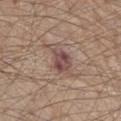notes — total-body-photography surveillance lesion; no biopsy | automated lesion analysis — a lesion area of about 7.5 mm², an eccentricity of roughly 0.8, and a shape-asymmetry score of about 0.35 (0 = symmetric); a lesion color around L≈48 a*≈18 b*≈20 in CIELAB and a lesion-to-skin contrast of about 8.5 (normalized; higher = more distinct); a border-irregularity index near 4/10 and peripheral color asymmetry of about 2 | image source — 15 mm crop, total-body photography | subject — male, in their 80s | anatomic site — the front of the torso | size — ≈4 mm.